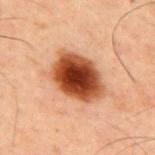| key | value |
|---|---|
| biopsy status | no biopsy performed (imaged during a skin exam) |
| body site | the upper back |
| lesion size | ~6 mm (longest diameter) |
| lighting | cross-polarized illumination |
| image | total-body-photography crop, ~15 mm field of view |
| patient | male, aged 58–62 |